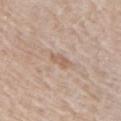Clinical impression:
Part of a total-body skin-imaging series; this lesion was reviewed on a skin check and was not flagged for biopsy.
Context:
A 15 mm crop from a total-body photograph taken for skin-cancer surveillance. The lesion is on the right upper arm. A male subject approximately 70 years of age. The lesion-visualizer software estimated a nevus-likeness score of about 0/100 and a detector confidence of about 100 out of 100 that the crop contains a lesion. This is a white-light tile.